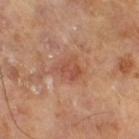Assessment:
The lesion was photographed on a routine skin check and not biopsied; there is no pathology result.
Image and clinical context:
The patient is a male in their mid-60s. About 3 mm across. A 15 mm crop from a total-body photograph taken for skin-cancer surveillance. The tile uses cross-polarized illumination. The total-body-photography lesion software estimated a footprint of about 5.5 mm². And it measured a lesion–skin lightness drop of about 7 and a normalized border contrast of about 5.5. The analysis additionally found a border-irregularity rating of about 4/10 and radial color variation of about 1. And it measured a nevus-likeness score of about 0/100 and lesion-presence confidence of about 100/100.